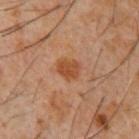{"biopsy_status": "not biopsied; imaged during a skin examination", "image": {"source": "total-body photography crop", "field_of_view_mm": 15}, "lighting": "cross-polarized", "automated_metrics": {"cielab_L": 45, "cielab_a": 24, "cielab_b": 34, "vs_skin_darker_L": 9.0, "vs_skin_contrast_norm": 8.0, "border_irregularity_0_10": 2.0, "color_variation_0_10": 3.0, "lesion_detection_confidence_0_100": 100}, "site": "chest", "patient": {"sex": "male", "age_approx": 60}}